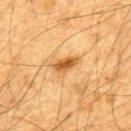On the upper back. Cropped from a total-body skin-imaging series; the visible field is about 15 mm. A male patient aged around 65. The tile uses cross-polarized illumination.